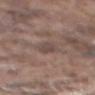follow-up: catalogued during a skin exam; not biopsied
subject: male, aged around 75
tile lighting: white-light
acquisition: total-body-photography crop, ~15 mm field of view
location: the mid back
image-analysis metrics: an area of roughly 5 mm² and an eccentricity of roughly 0.8; border irregularity of about 2 on a 0–10 scale, a color-variation rating of about 2/10, and a peripheral color-asymmetry measure near 0.5; lesion-presence confidence of about 85/100
size: ~3 mm (longest diameter)